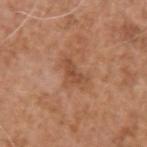Clinical impression: Recorded during total-body skin imaging; not selected for excision or biopsy. Background: A male patient, in their mid- to late 70s. On the left upper arm. The tile uses white-light illumination. A close-up tile cropped from a whole-body skin photograph, about 15 mm across. Longest diameter approximately 3.5 mm.